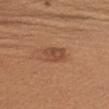Impression: Imaged during a routine full-body skin examination; the lesion was not biopsied and no histopathology is available. Image and clinical context: A female subject aged 43 to 47. An algorithmic analysis of the crop reported an area of roughly 6.5 mm², an eccentricity of roughly 0.55, and a shape-asymmetry score of about 0.2 (0 = symmetric). And it measured a lesion color around L≈48 a*≈23 b*≈32 in CIELAB, roughly 8 lightness units darker than nearby skin, and a normalized border contrast of about 6.5. The analysis additionally found border irregularity of about 2 on a 0–10 scale, a color-variation rating of about 3/10, and radial color variation of about 1. A close-up tile cropped from a whole-body skin photograph, about 15 mm across. The recorded lesion diameter is about 3 mm. Located on the right forearm.